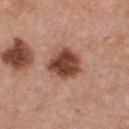Impression:
Imaged during a routine full-body skin examination; the lesion was not biopsied and no histopathology is available.
Background:
From the chest. A female subject, about 45 years old. The total-body-photography lesion software estimated a nevus-likeness score of about 65/100 and a lesion-detection confidence of about 100/100. Measured at roughly 4 mm in maximum diameter. Imaged with white-light lighting. Cropped from a whole-body photographic skin survey; the tile spans about 15 mm.